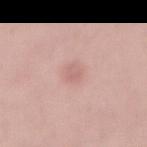Findings:
- notes · no biopsy performed (imaged during a skin exam)
- acquisition · ~15 mm crop, total-body skin-cancer survey
- subject · female, roughly 25 years of age
- automated lesion analysis · a lesion area of about 4 mm², an outline eccentricity of about 0.8 (0 = round, 1 = elongated), and a symmetry-axis asymmetry near 0.2
- illumination · white-light
- body site · the abdomen